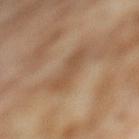Part of a total-body skin-imaging series; this lesion was reviewed on a skin check and was not flagged for biopsy.
This is a cross-polarized tile.
On the left thigh.
The patient is a female aged around 55.
About 4 mm across.
This image is a 15 mm lesion crop taken from a total-body photograph.